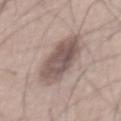notes: catalogued during a skin exam; not biopsied | patient: male, aged around 55 | image source: total-body-photography crop, ~15 mm field of view.